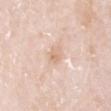The lesion was photographed on a routine skin check and not biopsied; there is no pathology result. A 15 mm close-up extracted from a 3D total-body photography capture. A male subject, in their 80s. Captured under white-light illumination. The lesion is located on the head or neck. Approximately 2.5 mm at its widest.